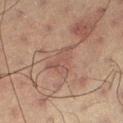workup: no biopsy performed (imaged during a skin exam); lesion diameter: ≈4 mm; patient: male, aged approximately 60; image: ~15 mm crop, total-body skin-cancer survey.